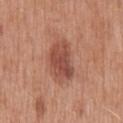Assessment:
No biopsy was performed on this lesion — it was imaged during a full skin examination and was not determined to be concerning.
Context:
From the mid back. The tile uses white-light illumination. A 15 mm close-up extracted from a 3D total-body photography capture. The total-body-photography lesion software estimated an eccentricity of roughly 0.85 and a symmetry-axis asymmetry near 0.2. And it measured an average lesion color of about L≈49 a*≈25 b*≈29 (CIELAB), a lesion–skin lightness drop of about 11, and a lesion-to-skin contrast of about 8 (normalized; higher = more distinct). The analysis additionally found a nevus-likeness score of about 70/100 and lesion-presence confidence of about 100/100. The recorded lesion diameter is about 5 mm. The patient is a male in their 70s.